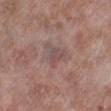{"biopsy_status": "not biopsied; imaged during a skin examination", "site": "right lower leg", "image": {"source": "total-body photography crop", "field_of_view_mm": 15}, "patient": {"sex": "male", "age_approx": 75}, "lesion_size": {"long_diameter_mm_approx": 3.0}, "automated_metrics": {"area_mm2_approx": 5.5, "eccentricity": 0.45, "border_irregularity_0_10": 5.0, "color_variation_0_10": 3.0, "peripheral_color_asymmetry": 1.0}}